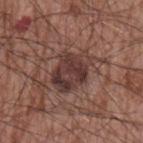Q: Is there a histopathology result?
A: total-body-photography surveillance lesion; no biopsy
Q: How was this image acquired?
A: ~15 mm tile from a whole-body skin photo
Q: What is the anatomic site?
A: the left upper arm
Q: Automated lesion metrics?
A: a mean CIELAB color near L≈37 a*≈19 b*≈20, about 10 CIELAB-L* units darker than the surrounding skin, and a normalized border contrast of about 9; border irregularity of about 2.5 on a 0–10 scale, a color-variation rating of about 6.5/10, and peripheral color asymmetry of about 2.5
Q: What are the patient's age and sex?
A: male, in their mid-60s
Q: Lesion size?
A: ≈5 mm